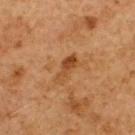Assessment: Imaged during a routine full-body skin examination; the lesion was not biopsied and no histopathology is available. Context: A male subject, in their 60s. A close-up tile cropped from a whole-body skin photograph, about 15 mm across. This is a cross-polarized tile. The recorded lesion diameter is about 4 mm. From the upper back.